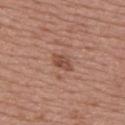follow-up: catalogued during a skin exam; not biopsied
imaging modality: total-body-photography crop, ~15 mm field of view
diameter: about 3 mm
anatomic site: the back
patient: female, roughly 50 years of age